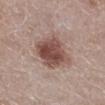Q: Was this lesion biopsied?
A: no biopsy performed (imaged during a skin exam)
Q: What did automated image analysis measure?
A: two-axis asymmetry of about 0.15; a border-irregularity index near 2/10 and a within-lesion color-variation index near 5/10; an automated nevus-likeness rating near 95 out of 100 and lesion-presence confidence of about 100/100
Q: What is the lesion's diameter?
A: ≈6 mm
Q: Who is the patient?
A: female, in their 50s
Q: How was this image acquired?
A: ~15 mm tile from a whole-body skin photo
Q: Where on the body is the lesion?
A: the right lower leg
Q: What lighting was used for the tile?
A: white-light illumination